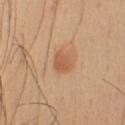Q: Was this lesion biopsied?
A: total-body-photography surveillance lesion; no biopsy
Q: What is the anatomic site?
A: the right upper arm
Q: How was the tile lit?
A: white-light illumination
Q: Lesion size?
A: ≈2.5 mm
Q: What kind of image is this?
A: ~15 mm tile from a whole-body skin photo
Q: What did automated image analysis measure?
A: a lesion area of about 5 mm² and a shape eccentricity near 0.6; a lesion color around L≈57 a*≈23 b*≈36 in CIELAB, about 9 CIELAB-L* units darker than the surrounding skin, and a normalized border contrast of about 6; border irregularity of about 1.5 on a 0–10 scale, a color-variation rating of about 2/10, and a peripheral color-asymmetry measure near 0.5
Q: What are the patient's age and sex?
A: male, aged 53 to 57